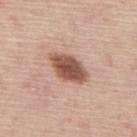Q: Was this lesion biopsied?
A: no biopsy performed (imaged during a skin exam)
Q: How was this image acquired?
A: ~15 mm tile from a whole-body skin photo
Q: Who is the patient?
A: male, in their mid-40s
Q: Lesion location?
A: the mid back